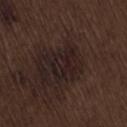Q: Is there a histopathology result?
A: no biopsy performed (imaged during a skin exam)